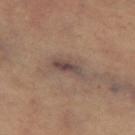Background: The lesion is on the leg. Imaged with cross-polarized lighting. Automated image analysis of the tile measured a within-lesion color-variation index near 4/10 and peripheral color asymmetry of about 1. The software also gave a classifier nevus-likeness of about 5/100. The patient is a female aged around 70. The lesion's longest dimension is about 4 mm. A 15 mm close-up extracted from a 3D total-body photography capture.